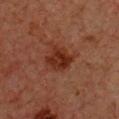The lesion was photographed on a routine skin check and not biopsied; there is no pathology result.
The lesion-visualizer software estimated a lesion area of about 8 mm² and two-axis asymmetry of about 0.2. The analysis additionally found a classifier nevus-likeness of about 60/100.
A female subject, aged approximately 40.
Located on the chest.
This image is a 15 mm lesion crop taken from a total-body photograph.